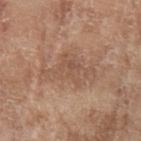follow-up — imaged on a skin check; not biopsied | anatomic site — the arm | lesion diameter — ≈6 mm | TBP lesion metrics — a lesion area of about 14 mm², an outline eccentricity of about 0.8 (0 = round, 1 = elongated), and two-axis asymmetry of about 0.45; a lesion–skin lightness drop of about 7 and a lesion-to-skin contrast of about 5 (normalized; higher = more distinct); a classifier nevus-likeness of about 0/100 and a lesion-detection confidence of about 100/100 | image — 15 mm crop, total-body photography | subject — female, roughly 75 years of age.